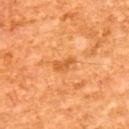The lesion was photographed on a routine skin check and not biopsied; there is no pathology result. The tile uses cross-polarized illumination. Cropped from a total-body skin-imaging series; the visible field is about 15 mm. The recorded lesion diameter is about 2.5 mm. Automated tile analysis of the lesion measured border irregularity of about 4 on a 0–10 scale, a color-variation rating of about 0.5/10, and radial color variation of about 0.5. A male patient aged 63 to 67. The lesion is on the upper back.